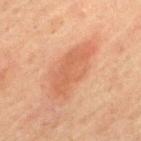| feature | finding |
|---|---|
| notes | imaged on a skin check; not biopsied |
| image-analysis metrics | an area of roughly 16 mm² and a shape-asymmetry score of about 0.25 (0 = symmetric); a mean CIELAB color near L≈50 a*≈22 b*≈31; a detector confidence of about 100 out of 100 that the crop contains a lesion |
| subject | male, about 60 years old |
| image | total-body-photography crop, ~15 mm field of view |
| lighting | cross-polarized |
| site | the back |
| size | ≈7 mm |